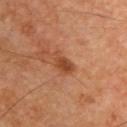Part of a total-body skin-imaging series; this lesion was reviewed on a skin check and was not flagged for biopsy. The lesion-visualizer software estimated an eccentricity of roughly 0.85 and two-axis asymmetry of about 0.25. The analysis additionally found a color-variation rating of about 2/10 and peripheral color asymmetry of about 0.5. It also reported a nevus-likeness score of about 15/100 and a lesion-detection confidence of about 100/100. This is a cross-polarized tile. The lesion's longest dimension is about 3 mm. A region of skin cropped from a whole-body photographic capture, roughly 15 mm wide. A male subject aged 58 to 62. From the upper back.